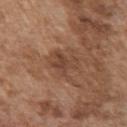Q: Was a biopsy performed?
A: catalogued during a skin exam; not biopsied